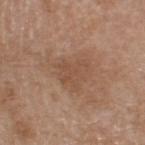Findings:
• follow-up — imaged on a skin check; not biopsied
• body site — the head or neck
• acquisition — ~15 mm tile from a whole-body skin photo
• illumination — white-light illumination
• subject — female, in their mid- to late 60s
• diameter — about 4 mm